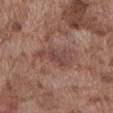{"biopsy_status": "not biopsied; imaged during a skin examination", "image": {"source": "total-body photography crop", "field_of_view_mm": 15}, "lesion_size": {"long_diameter_mm_approx": 5.0}, "patient": {"sex": "male", "age_approx": 75}, "site": "abdomen"}